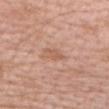This is a white-light tile. A female patient aged around 75. Automated tile analysis of the lesion measured an average lesion color of about L≈58 a*≈22 b*≈31 (CIELAB), a lesion–skin lightness drop of about 7, and a lesion-to-skin contrast of about 5.5 (normalized; higher = more distinct). It also reported a within-lesion color-variation index near 1/10 and radial color variation of about 0.5. The recorded lesion diameter is about 2.5 mm. Located on the head or neck. Cropped from a total-body skin-imaging series; the visible field is about 15 mm.